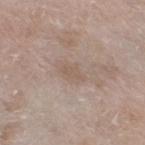{"biopsy_status": "not biopsied; imaged during a skin examination", "image": {"source": "total-body photography crop", "field_of_view_mm": 15}, "automated_metrics": {"eccentricity": 0.8, "cielab_L": 56, "cielab_a": 14, "cielab_b": 25, "vs_skin_darker_L": 6.0, "vs_skin_contrast_norm": 4.5, "border_irregularity_0_10": 3.5, "color_variation_0_10": 1.0, "peripheral_color_asymmetry": 0.0}, "patient": {"sex": "female", "age_approx": 75}, "site": "right thigh", "lighting": "white-light", "lesion_size": {"long_diameter_mm_approx": 3.0}}